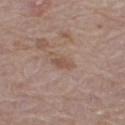A female patient, in their mid- to late 60s.
Imaged with white-light lighting.
Located on the left leg.
The recorded lesion diameter is about 3 mm.
This image is a 15 mm lesion crop taken from a total-body photograph.